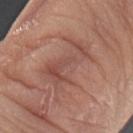Part of a total-body skin-imaging series; this lesion was reviewed on a skin check and was not flagged for biopsy. A female subject, roughly 65 years of age. From the left forearm. A 15 mm close-up extracted from a 3D total-body photography capture.